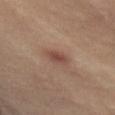Assessment:
Recorded during total-body skin imaging; not selected for excision or biopsy.
Background:
The lesion's longest dimension is about 2.5 mm. A lesion tile, about 15 mm wide, cut from a 3D total-body photograph. Automated image analysis of the tile measured a footprint of about 4 mm², an eccentricity of roughly 0.8, and a symmetry-axis asymmetry near 0.25. It also reported border irregularity of about 2 on a 0–10 scale, a color-variation rating of about 2/10, and a peripheral color-asymmetry measure near 0.5. The lesion is located on the front of the torso. The tile uses cross-polarized illumination. The subject is a female aged approximately 40.